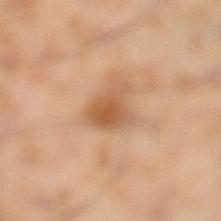biopsy status = no biopsy performed (imaged during a skin exam)
tile lighting = cross-polarized
image source = total-body-photography crop, ~15 mm field of view
diameter = about 3.5 mm
body site = the left lower leg
subject = male, approximately 55 years of age